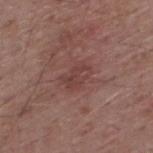Captured during whole-body skin photography for melanoma surveillance; the lesion was not biopsied. Automated image analysis of the tile measured a lesion area of about 6.5 mm² and an outline eccentricity of about 0.75 (0 = round, 1 = elongated). The analysis additionally found border irregularity of about 2.5 on a 0–10 scale, a within-lesion color-variation index near 3/10, and radial color variation of about 1. A lesion tile, about 15 mm wide, cut from a 3D total-body photograph. About 3.5 mm across. Imaged with white-light lighting. The subject is a male aged 53 to 57. The lesion is located on the upper back.